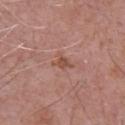biopsy status=total-body-photography surveillance lesion; no biopsy
size=≈2.5 mm
subject=male, about 70 years old
illumination=white-light illumination
body site=the chest
automated metrics=a border-irregularity index near 5/10, a color-variation rating of about 1.5/10, and peripheral color asymmetry of about 0.5; an automated nevus-likeness rating near 0 out of 100 and a lesion-detection confidence of about 100/100
acquisition=total-body-photography crop, ~15 mm field of view